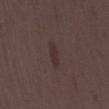Notes:
• biopsy status — catalogued during a skin exam; not biopsied
• subject — female, aged 33–37
• image source — ~15 mm tile from a whole-body skin photo
• location — the lower back
• tile lighting — white-light illumination